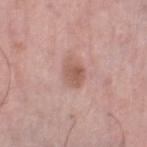This lesion was catalogued during total-body skin photography and was not selected for biopsy. Captured under white-light illumination. Located on the right lower leg. A roughly 15 mm field-of-view crop from a total-body skin photograph. A male patient aged 68–72. Automated tile analysis of the lesion measured a lesion color around L≈57 a*≈21 b*≈26 in CIELAB, roughly 10 lightness units darker than nearby skin, and a lesion-to-skin contrast of about 7 (normalized; higher = more distinct). It also reported a border-irregularity index near 1.5/10 and internal color variation of about 3 on a 0–10 scale.